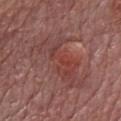| feature | finding |
|---|---|
| workup | imaged on a skin check; not biopsied |
| patient | male, aged around 75 |
| illumination | white-light illumination |
| anatomic site | the chest |
| lesion diameter | ≈8.5 mm |
| image | 15 mm crop, total-body photography |
| image-analysis metrics | roughly 6 lightness units darker than nearby skin; an automated nevus-likeness rating near 5 out of 100 and a lesion-detection confidence of about 95/100 |